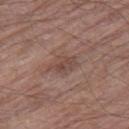Impression: The lesion was tiled from a total-body skin photograph and was not biopsied. Background: Captured under white-light illumination. Cropped from a whole-body photographic skin survey; the tile spans about 15 mm. A male patient, approximately 80 years of age. On the right thigh. About 3.5 mm across.